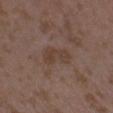Imaged during a routine full-body skin examination; the lesion was not biopsied and no histopathology is available. A roughly 15 mm field-of-view crop from a total-body skin photograph. A female patient approximately 35 years of age. The recorded lesion diameter is about 4 mm. This is a white-light tile. The lesion is located on the right forearm.